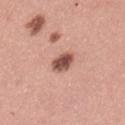Recorded during total-body skin imaging; not selected for excision or biopsy. Cropped from a whole-body photographic skin survey; the tile spans about 15 mm. The lesion is located on the leg. A female subject roughly 35 years of age.